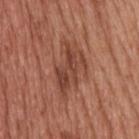Clinical impression:
The lesion was tiled from a total-body skin photograph and was not biopsied.
Context:
A male subject, about 60 years old. A lesion tile, about 15 mm wide, cut from a 3D total-body photograph. The lesion is located on the upper back. Longest diameter approximately 7 mm.